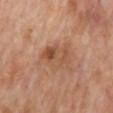Clinical impression: Imaged during a routine full-body skin examination; the lesion was not biopsied and no histopathology is available. Context: A male patient, roughly 65 years of age. About 4.5 mm across. Located on the mid back. A roughly 15 mm field-of-view crop from a total-body skin photograph.